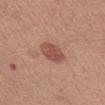Findings:
- workup: imaged on a skin check; not biopsied
- patient: male, aged approximately 40
- imaging modality: 15 mm crop, total-body photography
- body site: the left upper arm
- image-analysis metrics: an area of roughly 7 mm², a shape eccentricity near 0.6, and a symmetry-axis asymmetry near 0.15; an average lesion color of about L≈51 a*≈24 b*≈27 (CIELAB), roughly 10 lightness units darker than nearby skin, and a lesion-to-skin contrast of about 7.5 (normalized; higher = more distinct)
- tile lighting: white-light